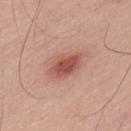Clinical impression:
This lesion was catalogued during total-body skin photography and was not selected for biopsy.
Image and clinical context:
This image is a 15 mm lesion crop taken from a total-body photograph. The tile uses white-light illumination. About 5 mm across. The patient is a male aged around 50. The lesion is located on the lower back.